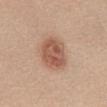biopsy status: imaged on a skin check; not biopsied | diameter: ~4 mm (longest diameter) | patient: female, in their mid-50s | image source: ~15 mm tile from a whole-body skin photo | tile lighting: white-light illumination | location: the abdomen.